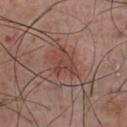Q: Was a biopsy performed?
A: total-body-photography surveillance lesion; no biopsy
Q: What is the imaging modality?
A: ~15 mm tile from a whole-body skin photo
Q: Lesion location?
A: the chest
Q: What lighting was used for the tile?
A: white-light
Q: Who is the patient?
A: male, about 55 years old